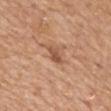biopsy status: no biopsy performed (imaged during a skin exam) | patient: female, aged around 70 | site: the mid back | imaging modality: ~15 mm crop, total-body skin-cancer survey | lesion size: about 2.5 mm | image-analysis metrics: an average lesion color of about L≈53 a*≈23 b*≈33 (CIELAB), a lesion–skin lightness drop of about 11, and a normalized lesion–skin contrast near 7.5; border irregularity of about 3.5 on a 0–10 scale, a color-variation rating of about 2/10, and a peripheral color-asymmetry measure near 0.5; an automated nevus-likeness rating near 10 out of 100 and a lesion-detection confidence of about 100/100 | illumination: white-light.